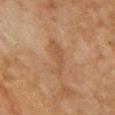| feature | finding |
|---|---|
| notes | total-body-photography surveillance lesion; no biopsy |
| site | the chest |
| image | total-body-photography crop, ~15 mm field of view |
| lighting | cross-polarized |
| patient | female, about 60 years old |
| TBP lesion metrics | an area of roughly 7.5 mm² and a symmetry-axis asymmetry near 0.4; a lesion-to-skin contrast of about 4.5 (normalized; higher = more distinct); internal color variation of about 1 on a 0–10 scale |